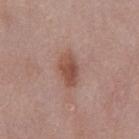The lesion was photographed on a routine skin check and not biopsied; there is no pathology result.